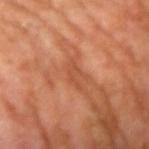location = the right upper arm
lighting = cross-polarized illumination
subject = male, in their mid-50s
image = 15 mm crop, total-body photography
lesion diameter = about 3 mm